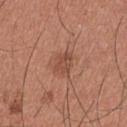Impression:
This lesion was catalogued during total-body skin photography and was not selected for biopsy.
Background:
Cropped from a whole-body photographic skin survey; the tile spans about 15 mm. A male subject approximately 35 years of age. Automated tile analysis of the lesion measured a shape eccentricity near 0.65 and a symmetry-axis asymmetry near 0.2. And it measured a border-irregularity index near 2.5/10, internal color variation of about 2.5 on a 0–10 scale, and radial color variation of about 1. On the right upper arm. The tile uses white-light illumination.